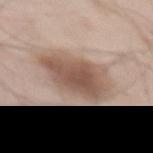Findings:
* notes: imaged on a skin check; not biopsied
* lesion diameter: ~6.5 mm (longest diameter)
* image source: ~15 mm tile from a whole-body skin photo
* patient: male, approximately 55 years of age
* anatomic site: the abdomen
* tile lighting: white-light illumination